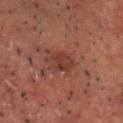biopsy status = catalogued during a skin exam; not biopsied
image source = ~15 mm tile from a whole-body skin photo
patient = male, aged 48 to 52
illumination = cross-polarized illumination
body site = the head or neck
size = ≈3.5 mm